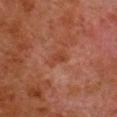Recorded during total-body skin imaging; not selected for excision or biopsy. A 15 mm close-up tile from a total-body photography series done for melanoma screening. The lesion-visualizer software estimated a border-irregularity index near 4/10. And it measured an automated nevus-likeness rating near 0 out of 100 and a detector confidence of about 100 out of 100 that the crop contains a lesion. Longest diameter approximately 2.5 mm. A male subject, aged around 80. The lesion is on the right lower leg. Captured under cross-polarized illumination.